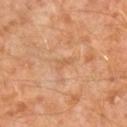notes — no biopsy performed (imaged during a skin exam)
anatomic site — the leg
lesion diameter — ≈2.5 mm
patient — male, approximately 30 years of age
automated metrics — an eccentricity of roughly 0.9 and a shape-asymmetry score of about 0.4 (0 = symmetric); a lesion color around L≈58 a*≈22 b*≈37 in CIELAB, a lesion–skin lightness drop of about 6, and a lesion-to-skin contrast of about 4.5 (normalized; higher = more distinct)
image — ~15 mm tile from a whole-body skin photo